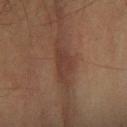No biopsy was performed on this lesion — it was imaged during a full skin examination and was not determined to be concerning.
A male patient, aged 58 to 62.
About 5.5 mm across.
The tile uses cross-polarized illumination.
A 15 mm close-up tile from a total-body photography series done for melanoma screening.
On the right forearm.